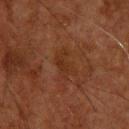notes = catalogued during a skin exam; not biopsied.